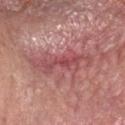Recorded during total-body skin imaging; not selected for excision or biopsy.
A region of skin cropped from a whole-body photographic capture, roughly 15 mm wide.
A male subject, about 80 years old.
The lesion is on the head or neck.
The lesion-visualizer software estimated a lesion color around L≈51 a*≈29 b*≈21 in CIELAB and a lesion–skin lightness drop of about 9. The analysis additionally found border irregularity of about 8 on a 0–10 scale. It also reported an automated nevus-likeness rating near 0 out of 100 and a detector confidence of about 55 out of 100 that the crop contains a lesion.
Longest diameter approximately 7.5 mm.